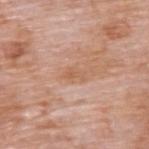Part of a total-body skin-imaging series; this lesion was reviewed on a skin check and was not flagged for biopsy.